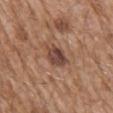Imaged during a routine full-body skin examination; the lesion was not biopsied and no histopathology is available.
Automated image analysis of the tile measured an average lesion color of about L≈44 a*≈20 b*≈25 (CIELAB), roughly 12 lightness units darker than nearby skin, and a normalized border contrast of about 9.5. The analysis additionally found peripheral color asymmetry of about 2. It also reported an automated nevus-likeness rating near 0 out of 100.
About 3 mm across.
A 15 mm crop from a total-body photograph taken for skin-cancer surveillance.
A male subject, approximately 60 years of age.
Located on the left upper arm.